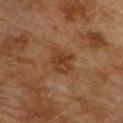Clinical impression: Recorded during total-body skin imaging; not selected for excision or biopsy. Acquisition and patient details: Imaged with cross-polarized lighting. A close-up tile cropped from a whole-body skin photograph, about 15 mm across. The lesion is on the front of the torso. Measured at roughly 3 mm in maximum diameter. A male subject aged around 75.